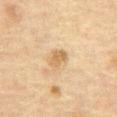workup = imaged on a skin check; not biopsied
imaging modality = total-body-photography crop, ~15 mm field of view
subject = male, aged 73 to 77
anatomic site = the front of the torso
lighting = cross-polarized illumination
lesion size = ≈2.5 mm